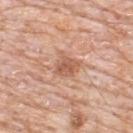Findings:
– follow-up — total-body-photography surveillance lesion; no biopsy
– location — the upper back
– diameter — about 3 mm
– acquisition — ~15 mm tile from a whole-body skin photo
– lighting — white-light
– subject — male, aged approximately 80